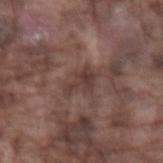No biopsy was performed on this lesion — it was imaged during a full skin examination and was not determined to be concerning. Automated tile analysis of the lesion measured an area of roughly 5 mm² and two-axis asymmetry of about 0.45. The software also gave a lesion color around L≈38 a*≈17 b*≈20 in CIELAB, roughly 8 lightness units darker than nearby skin, and a normalized lesion–skin contrast near 7. The software also gave a border-irregularity rating of about 5.5/10, internal color variation of about 3 on a 0–10 scale, and radial color variation of about 1. It also reported a lesion-detection confidence of about 70/100. From the left forearm. The patient is a male in their mid- to late 70s. A 15 mm crop from a total-body photograph taken for skin-cancer surveillance.